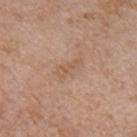notes = no biopsy performed (imaged during a skin exam); lighting = white-light illumination; subject = male, in their mid-60s; body site = the chest; image source = total-body-photography crop, ~15 mm field of view.